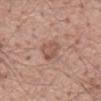The lesion was tiled from a total-body skin photograph and was not biopsied. A 15 mm crop from a total-body photograph taken for skin-cancer surveillance. Automated image analysis of the tile measured an area of roughly 5.5 mm², an eccentricity of roughly 0.8, and a shape-asymmetry score of about 0.3 (0 = symmetric). It also reported a lesion-detection confidence of about 100/100. The lesion's longest dimension is about 3.5 mm. Captured under white-light illumination. A male patient, aged 53 to 57. The lesion is on the mid back.